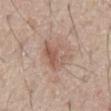follow-up = no biopsy performed (imaged during a skin exam) | anatomic site = the abdomen | acquisition = ~15 mm tile from a whole-body skin photo | subject = male, aged 63–67.